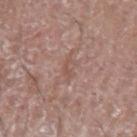Notes:
- workup · total-body-photography surveillance lesion; no biopsy
- automated lesion analysis · a footprint of about 3.5 mm², a shape eccentricity near 0.9, and two-axis asymmetry of about 0.3; a normalized lesion–skin contrast near 4.5; a border-irregularity rating of about 4/10, a within-lesion color-variation index near 2.5/10, and peripheral color asymmetry of about 0.5; a detector confidence of about 85 out of 100 that the crop contains a lesion
- lesion diameter · about 3.5 mm
- body site · the left upper arm
- lighting · white-light
- image source · ~15 mm crop, total-body skin-cancer survey
- patient · male, roughly 65 years of age